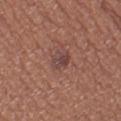Impression: This lesion was catalogued during total-body skin photography and was not selected for biopsy. Clinical summary: A lesion tile, about 15 mm wide, cut from a 3D total-body photograph. About 2.5 mm across. A female subject aged approximately 40. The lesion is located on the left thigh. This is a white-light tile.